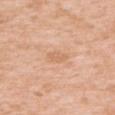Background:
Approximately 3 mm at its widest. The subject is a female aged 38–42. Captured under white-light illumination. A lesion tile, about 15 mm wide, cut from a 3D total-body photograph. From the back. An algorithmic analysis of the crop reported a lesion color around L≈66 a*≈21 b*≈35 in CIELAB, a lesion–skin lightness drop of about 7, and a lesion-to-skin contrast of about 4.5 (normalized; higher = more distinct). It also reported a border-irregularity index near 2/10, a color-variation rating of about 2/10, and radial color variation of about 0.5. It also reported a nevus-likeness score of about 0/100 and a detector confidence of about 100 out of 100 that the crop contains a lesion.